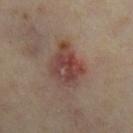Notes:
– biopsy status — total-body-photography surveillance lesion; no biopsy
– imaging modality — ~15 mm tile from a whole-body skin photo
– subject — female, about 35 years old
– lesion size — ≈5 mm
– image-analysis metrics — an average lesion color of about L≈40 a*≈20 b*≈22 (CIELAB) and a normalized border contrast of about 8.5; an automated nevus-likeness rating near 25 out of 100 and lesion-presence confidence of about 100/100
– anatomic site — the right lower leg
– lighting — cross-polarized